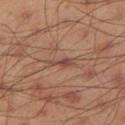Impression:
The lesion was photographed on a routine skin check and not biopsied; there is no pathology result.